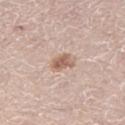Part of a total-body skin-imaging series; this lesion was reviewed on a skin check and was not flagged for biopsy.
An algorithmic analysis of the crop reported an area of roughly 4.5 mm², an outline eccentricity of about 0.7 (0 = round, 1 = elongated), and two-axis asymmetry of about 0.25. And it measured a lesion color around L≈60 a*≈18 b*≈27 in CIELAB and about 12 CIELAB-L* units darker than the surrounding skin. It also reported border irregularity of about 2.5 on a 0–10 scale, a color-variation rating of about 3.5/10, and peripheral color asymmetry of about 1.
This image is a 15 mm lesion crop taken from a total-body photograph.
A female subject, aged approximately 45.
From the left thigh.
The tile uses white-light illumination.